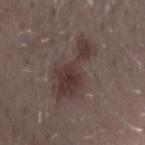No biopsy was performed on this lesion — it was imaged during a full skin examination and was not determined to be concerning.
The lesion is located on the right forearm.
The lesion-visualizer software estimated an eccentricity of roughly 0.95 and a shape-asymmetry score of about 0.55 (0 = symmetric).
A roughly 15 mm field-of-view crop from a total-body skin photograph.
The lesion's longest dimension is about 8 mm.
A male patient aged around 30.